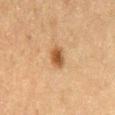workup: no biopsy performed (imaged during a skin exam)
imaging modality: ~15 mm crop, total-body skin-cancer survey
automated metrics: an eccentricity of roughly 0.6 and two-axis asymmetry of about 0.15; a lesion color around L≈44 a*≈18 b*≈33 in CIELAB, about 11 CIELAB-L* units darker than the surrounding skin, and a lesion-to-skin contrast of about 9 (normalized; higher = more distinct); a border-irregularity index near 1.5/10, internal color variation of about 3.5 on a 0–10 scale, and peripheral color asymmetry of about 1
location: the lower back
illumination: cross-polarized
diameter: ~2.5 mm (longest diameter)
subject: male, aged around 75